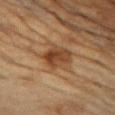  patient:
    sex: female
    age_approx: 80
  lighting: cross-polarized
  site: chest
  image:
    source: total-body photography crop
    field_of_view_mm: 15